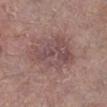workup: total-body-photography surveillance lesion; no biopsy | tile lighting: white-light | image: ~15 mm crop, total-body skin-cancer survey | size: ≈6.5 mm | anatomic site: the leg | subject: male, roughly 55 years of age.